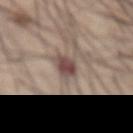No biopsy was performed on this lesion — it was imaged during a full skin examination and was not determined to be concerning.
About 3 mm across.
From the abdomen.
A 15 mm crop from a total-body photograph taken for skin-cancer surveillance.
The subject is a male aged around 60.